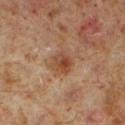Part of a total-body skin-imaging series; this lesion was reviewed on a skin check and was not flagged for biopsy.
Captured under cross-polarized illumination.
Cropped from a whole-body photographic skin survey; the tile spans about 15 mm.
A male subject, about 60 years old.
Automated image analysis of the tile measured an outline eccentricity of about 0.4 (0 = round, 1 = elongated) and a symmetry-axis asymmetry near 0.2. And it measured an average lesion color of about L≈43 a*≈21 b*≈31 (CIELAB).
Located on the left lower leg.
The recorded lesion diameter is about 3 mm.